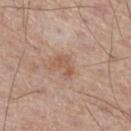Clinical impression: The lesion was photographed on a routine skin check and not biopsied; there is no pathology result. Background: The tile uses white-light illumination. Automated tile analysis of the lesion measured an automated nevus-likeness rating near 0 out of 100 and lesion-presence confidence of about 100/100. The subject is a male aged approximately 60. From the leg. About 3 mm across. A roughly 15 mm field-of-view crop from a total-body skin photograph.